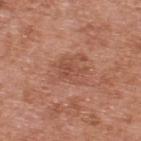| key | value |
|---|---|
| biopsy status | no biopsy performed (imaged during a skin exam) |
| lighting | white-light |
| anatomic site | the upper back |
| patient | male, approximately 70 years of age |
| acquisition | total-body-photography crop, ~15 mm field of view |
| size | about 4.5 mm |
| image-analysis metrics | a shape eccentricity near 0.6 and a shape-asymmetry score of about 0.3 (0 = symmetric); a mean CIELAB color near L≈51 a*≈24 b*≈30, about 7 CIELAB-L* units darker than the surrounding skin, and a normalized lesion–skin contrast near 5; border irregularity of about 4 on a 0–10 scale, internal color variation of about 3.5 on a 0–10 scale, and radial color variation of about 1.5; a classifier nevus-likeness of about 0/100 and a lesion-detection confidence of about 100/100 |